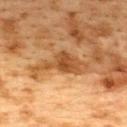{"biopsy_status": "not biopsied; imaged during a skin examination", "lesion_size": {"long_diameter_mm_approx": 6.5}, "automated_metrics": {"eccentricity": 0.9, "shape_asymmetry": 0.55, "cielab_L": 42, "cielab_a": 20, "cielab_b": 35, "vs_skin_darker_L": 11.0, "vs_skin_contrast_norm": 8.5, "nevus_likeness_0_100": 0}, "patient": {"sex": "female", "age_approx": 40}, "image": {"source": "total-body photography crop", "field_of_view_mm": 15}, "site": "upper back", "lighting": "cross-polarized"}